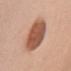{"biopsy_status": "not biopsied; imaged during a skin examination", "patient": {"sex": "female", "age_approx": 40}, "site": "left upper arm", "image": {"source": "total-body photography crop", "field_of_view_mm": 15}, "lesion_size": {"long_diameter_mm_approx": 7.5}, "lighting": "white-light"}